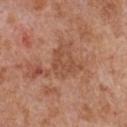Notes:
– biopsy status: total-body-photography surveillance lesion; no biopsy
– tile lighting: white-light
– imaging modality: 15 mm crop, total-body photography
– size: about 3.5 mm
– subject: male, in their mid- to late 60s
– site: the front of the torso